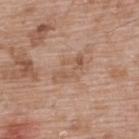Q: Was a biopsy performed?
A: no biopsy performed (imaged during a skin exam)
Q: What lighting was used for the tile?
A: white-light illumination
Q: How was this image acquired?
A: 15 mm crop, total-body photography
Q: What are the patient's age and sex?
A: male, in their 50s
Q: Lesion size?
A: about 4.5 mm
Q: What is the anatomic site?
A: the upper back